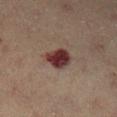<record>
  <biopsy_status>not biopsied; imaged during a skin examination</biopsy_status>
  <image>
    <source>total-body photography crop</source>
    <field_of_view_mm>15</field_of_view_mm>
  </image>
  <site>left lower leg</site>
  <lighting>cross-polarized</lighting>
  <lesion_size>
    <long_diameter_mm_approx>3.5</long_diameter_mm_approx>
  </lesion_size>
  <patient>
    <sex>male</sex>
    <age_approx>60</age_approx>
  </patient>
</record>